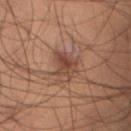About 4 mm across. This is a cross-polarized tile. The subject is a male approximately 50 years of age. On the right thigh. A 15 mm close-up tile from a total-body photography series done for melanoma screening.